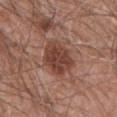The lesion was photographed on a routine skin check and not biopsied; there is no pathology result.
About 5 mm across.
The patient is a male aged approximately 60.
This image is a 15 mm lesion crop taken from a total-body photograph.
On the lower back.